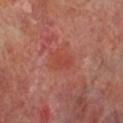Assessment:
This lesion was catalogued during total-body skin photography and was not selected for biopsy.
Image and clinical context:
The lesion's longest dimension is about 3 mm. The patient is a male in their 70s. A roughly 15 mm field-of-view crop from a total-body skin photograph. The lesion is located on the leg.